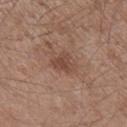This lesion was catalogued during total-body skin photography and was not selected for biopsy.
The lesion is on the right lower leg.
Captured under white-light illumination.
A 15 mm close-up tile from a total-body photography series done for melanoma screening.
An algorithmic analysis of the crop reported an outline eccentricity of about 0.6 (0 = round, 1 = elongated) and two-axis asymmetry of about 0.45. The analysis additionally found border irregularity of about 4 on a 0–10 scale, a color-variation rating of about 2.5/10, and a peripheral color-asymmetry measure near 1. The software also gave a nevus-likeness score of about 0/100 and a detector confidence of about 100 out of 100 that the crop contains a lesion.
A male subject, approximately 55 years of age.